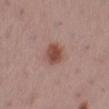No biopsy was performed on this lesion — it was imaged during a full skin examination and was not determined to be concerning.
A female subject aged approximately 55.
Located on the left lower leg.
The recorded lesion diameter is about 3 mm.
Captured under white-light illumination.
A close-up tile cropped from a whole-body skin photograph, about 15 mm across.